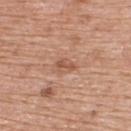{
  "biopsy_status": "not biopsied; imaged during a skin examination",
  "site": "upper back",
  "automated_metrics": {
    "area_mm2_approx": 2.5,
    "shape_asymmetry": 0.4,
    "border_irregularity_0_10": 4.5,
    "color_variation_0_10": 0.0
  },
  "lighting": "white-light",
  "image": {
    "source": "total-body photography crop",
    "field_of_view_mm": 15
  },
  "patient": {
    "sex": "male",
    "age_approx": 60
  },
  "lesion_size": {
    "long_diameter_mm_approx": 2.5
  }
}